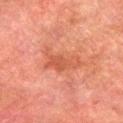| field | value |
|---|---|
| follow-up | no biopsy performed (imaged during a skin exam) |
| size | ≈5.5 mm |
| tile lighting | cross-polarized illumination |
| anatomic site | the left lower leg |
| image source | 15 mm crop, total-body photography |
| patient | male, about 75 years old |